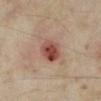Recorded during total-body skin imaging; not selected for excision or biopsy. The lesion's longest dimension is about 3.5 mm. A female patient, in their mid-30s. Automated tile analysis of the lesion measured an average lesion color of about L≈45 a*≈23 b*≈25 (CIELAB), roughly 13 lightness units darker than nearby skin, and a normalized border contrast of about 10. The software also gave border irregularity of about 2 on a 0–10 scale, internal color variation of about 6.5 on a 0–10 scale, and radial color variation of about 2. It also reported an automated nevus-likeness rating near 80 out of 100 and a detector confidence of about 100 out of 100 that the crop contains a lesion. Imaged with cross-polarized lighting. On the right lower leg. Cropped from a whole-body photographic skin survey; the tile spans about 15 mm.